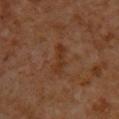workup: no biopsy performed (imaged during a skin exam)
location: the back
subject: male, aged around 60
illumination: cross-polarized
image: ~15 mm crop, total-body skin-cancer survey